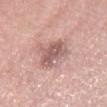Assessment: No biopsy was performed on this lesion — it was imaged during a full skin examination and was not determined to be concerning. Context: Cropped from a total-body skin-imaging series; the visible field is about 15 mm. The total-body-photography lesion software estimated a lesion color around L≈58 a*≈21 b*≈22 in CIELAB, roughly 12 lightness units darker than nearby skin, and a normalized border contrast of about 7.5. It also reported an automated nevus-likeness rating near 5 out of 100. The subject is a female roughly 40 years of age. Located on the leg. Captured under white-light illumination. About 5 mm across.